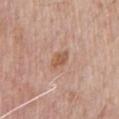Assessment: The lesion was tiled from a total-body skin photograph and was not biopsied. Acquisition and patient details: The patient is a male in their 70s. The tile uses white-light illumination. A 15 mm close-up extracted from a 3D total-body photography capture. From the chest. Longest diameter approximately 2.5 mm.